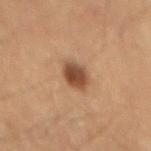No biopsy was performed on this lesion — it was imaged during a full skin examination and was not determined to be concerning. A roughly 15 mm field-of-view crop from a total-body skin photograph. The lesion's longest dimension is about 3.5 mm. A male subject, roughly 60 years of age. The lesion-visualizer software estimated a footprint of about 7 mm² and a symmetry-axis asymmetry near 0.15. The software also gave an average lesion color of about L≈41 a*≈18 b*≈29 (CIELAB), a lesion–skin lightness drop of about 13, and a normalized lesion–skin contrast near 10. And it measured a border-irregularity index near 2/10 and internal color variation of about 4 on a 0–10 scale. Imaged with cross-polarized lighting.